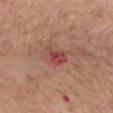Imaged during a routine full-body skin examination; the lesion was not biopsied and no histopathology is available. The tile uses white-light illumination. A lesion tile, about 15 mm wide, cut from a 3D total-body photograph. The patient is a female in their mid- to late 60s. The lesion is located on the mid back.